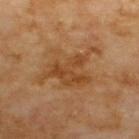Impression: The lesion was photographed on a routine skin check and not biopsied; there is no pathology result. Context: Located on the upper back. Imaged with cross-polarized lighting. Cropped from a whole-body photographic skin survey; the tile spans about 15 mm. An algorithmic analysis of the crop reported a border-irregularity rating of about 9/10 and peripheral color asymmetry of about 1.5. It also reported a nevus-likeness score of about 5/100. The recorded lesion diameter is about 7.5 mm. A male patient approximately 70 years of age.